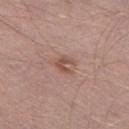Clinical impression: Recorded during total-body skin imaging; not selected for excision or biopsy. Context: A male patient aged around 55. On the left thigh. An algorithmic analysis of the crop reported a mean CIELAB color near L≈51 a*≈20 b*≈25. And it measured a nevus-likeness score of about 30/100 and a lesion-detection confidence of about 100/100. Imaged with white-light lighting. The recorded lesion diameter is about 2.5 mm. A 15 mm close-up extracted from a 3D total-body photography capture.